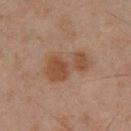The lesion was photographed on a routine skin check and not biopsied; there is no pathology result.
A male patient, in their mid-40s.
Located on the left lower leg.
Cropped from a whole-body photographic skin survey; the tile spans about 15 mm.
This is a cross-polarized tile.